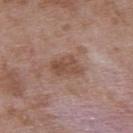Imaged during a routine full-body skin examination; the lesion was not biopsied and no histopathology is available.
Measured at roughly 3.5 mm in maximum diameter.
The lesion is located on the upper back.
A 15 mm close-up tile from a total-body photography series done for melanoma screening.
The patient is a male roughly 50 years of age.
Imaged with white-light lighting.
An algorithmic analysis of the crop reported an average lesion color of about L≈49 a*≈19 b*≈27 (CIELAB) and about 9 CIELAB-L* units darker than the surrounding skin. The analysis additionally found internal color variation of about 3 on a 0–10 scale and a peripheral color-asymmetry measure near 1.5. The software also gave an automated nevus-likeness rating near 0 out of 100 and a detector confidence of about 100 out of 100 that the crop contains a lesion.